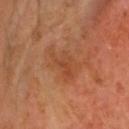Q: Is there a histopathology result?
A: no biopsy performed (imaged during a skin exam)
Q: Illumination type?
A: cross-polarized
Q: Patient demographics?
A: male, roughly 65 years of age
Q: What is the imaging modality?
A: ~15 mm tile from a whole-body skin photo
Q: What is the lesion's diameter?
A: ≈4 mm